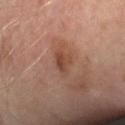<record>
  <biopsy_status>not biopsied; imaged during a skin examination</biopsy_status>
  <patient>
    <sex>male</sex>
    <age_approx>50</age_approx>
  </patient>
  <image>
    <source>total-body photography crop</source>
    <field_of_view_mm>15</field_of_view_mm>
  </image>
  <site>arm</site>
</record>